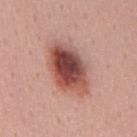image source=~15 mm crop, total-body skin-cancer survey; patient=female, aged around 45; illumination=white-light; location=the mid back.